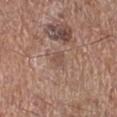Impression: This lesion was catalogued during total-body skin photography and was not selected for biopsy. Clinical summary: About 1.5 mm across. A male subject aged 68–72. The lesion is located on the leg. Cropped from a whole-body photographic skin survey; the tile spans about 15 mm. The tile uses white-light illumination.